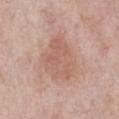notes = imaged on a skin check; not biopsied
image-analysis metrics = border irregularity of about 3.5 on a 0–10 scale, a within-lesion color-variation index near 3/10, and radial color variation of about 1
lesion diameter = ~6 mm (longest diameter)
lighting = white-light illumination
acquisition = total-body-photography crop, ~15 mm field of view
body site = the chest
patient = male, aged approximately 60